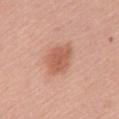Findings:
– workup: catalogued during a skin exam; not biopsied
– lighting: white-light illumination
– location: the chest
– image source: 15 mm crop, total-body photography
– diameter: ≈4 mm
– patient: female, in their mid- to late 60s